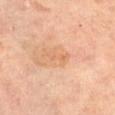Findings:
* notes · no biopsy performed (imaged during a skin exam)
* illumination · cross-polarized illumination
* anatomic site · the abdomen
* imaging modality · total-body-photography crop, ~15 mm field of view
* TBP lesion metrics · a mean CIELAB color near L≈65 a*≈22 b*≈37, a lesion–skin lightness drop of about 6, and a normalized lesion–skin contrast near 5; a nevus-likeness score of about 0/100 and a detector confidence of about 100 out of 100 that the crop contains a lesion
* lesion diameter · ≈3.5 mm
* subject · female, aged 63–67